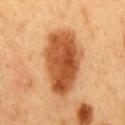Impression:
Part of a total-body skin-imaging series; this lesion was reviewed on a skin check and was not flagged for biopsy.
Image and clinical context:
Cropped from a total-body skin-imaging series; the visible field is about 15 mm. The lesion is on the mid back. The patient is a male aged around 50. The tile uses cross-polarized illumination.